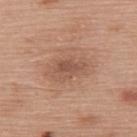The tile uses white-light illumination.
About 3.5 mm across.
This image is a 15 mm lesion crop taken from a total-body photograph.
From the upper back.
A female subject aged 58 to 62.